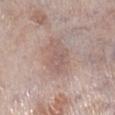Captured during whole-body skin photography for melanoma surveillance; the lesion was not biopsied.
A male patient about 60 years old.
The tile uses white-light illumination.
On the right lower leg.
Cropped from a total-body skin-imaging series; the visible field is about 15 mm.
Measured at roughly 5 mm in maximum diameter.
The total-body-photography lesion software estimated an automated nevus-likeness rating near 0 out of 100 and a detector confidence of about 100 out of 100 that the crop contains a lesion.